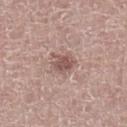{"site": "left lower leg", "patient": {"sex": "male", "age_approx": 65}, "lighting": "white-light", "image": {"source": "total-body photography crop", "field_of_view_mm": 15}, "automated_metrics": {"area_mm2_approx": 5.5, "eccentricity": 0.55, "shape_asymmetry": 0.25, "cielab_L": 53, "cielab_a": 19, "cielab_b": 22, "vs_skin_darker_L": 11.0, "vs_skin_contrast_norm": 7.5, "color_variation_0_10": 2.0, "nevus_likeness_0_100": 55, "lesion_detection_confidence_0_100": 100}}